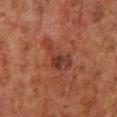Captured during whole-body skin photography for melanoma surveillance; the lesion was not biopsied.
Approximately 4.5 mm at its widest.
The lesion is on the left lower leg.
A 15 mm close-up tile from a total-body photography series done for melanoma screening.
Automated image analysis of the tile measured a border-irregularity index near 5.5/10 and a within-lesion color-variation index near 4.5/10. The software also gave a nevus-likeness score of about 5/100 and a detector confidence of about 100 out of 100 that the crop contains a lesion.
A male patient, in their 80s.